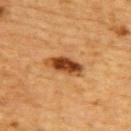{"biopsy_status": "not biopsied; imaged during a skin examination", "patient": {"sex": "male", "age_approx": "85+"}, "site": "upper back", "lesion_size": {"long_diameter_mm_approx": 4.5}, "automated_metrics": {"area_mm2_approx": 7.5, "eccentricity": 0.9, "shape_asymmetry": 0.2, "cielab_L": 39, "cielab_a": 23, "cielab_b": 37, "vs_skin_darker_L": 15.0, "vs_skin_contrast_norm": 12.0, "border_irregularity_0_10": 2.5, "color_variation_0_10": 6.0, "peripheral_color_asymmetry": 2.0}, "image": {"source": "total-body photography crop", "field_of_view_mm": 15}, "lighting": "cross-polarized"}